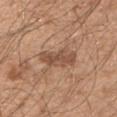| feature | finding |
|---|---|
| follow-up | catalogued during a skin exam; not biopsied |
| body site | the left upper arm |
| subject | male, aged around 50 |
| imaging modality | total-body-photography crop, ~15 mm field of view |
| lesion diameter | about 5 mm |
| illumination | white-light |